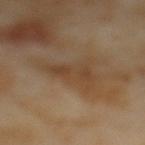Part of a total-body skin-imaging series; this lesion was reviewed on a skin check and was not flagged for biopsy. About 5.5 mm across. A female subject, aged 53 to 57. This image is a 15 mm lesion crop taken from a total-body photograph. The lesion is on the mid back.